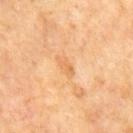workup: imaged on a skin check; not biopsied
site: the chest
subject: male, aged 63–67
imaging modality: 15 mm crop, total-body photography